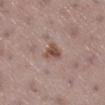Q: How large is the lesion?
A: ~2.5 mm (longest diameter)
Q: How was the tile lit?
A: white-light
Q: Who is the patient?
A: female, aged 53 to 57
Q: Automated lesion metrics?
A: a shape eccentricity near 0.6 and a symmetry-axis asymmetry near 0.4; an average lesion color of about L≈50 a*≈19 b*≈25 (CIELAB), roughly 11 lightness units darker than nearby skin, and a normalized lesion–skin contrast near 8.5; a border-irregularity rating of about 3.5/10 and radial color variation of about 1; a classifier nevus-likeness of about 85/100
Q: Where on the body is the lesion?
A: the right lower leg
Q: How was this image acquired?
A: 15 mm crop, total-body photography